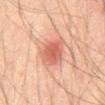Impression:
Imaged during a routine full-body skin examination; the lesion was not biopsied and no histopathology is available.
Background:
A 15 mm close-up tile from a total-body photography series done for melanoma screening. The patient is a male in their mid-40s. From the abdomen.